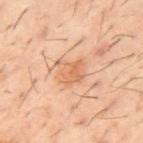biopsy status = no biopsy performed (imaged during a skin exam)
TBP lesion metrics = an automated nevus-likeness rating near 20 out of 100 and lesion-presence confidence of about 100/100
subject = male, in their 60s
acquisition = total-body-photography crop, ~15 mm field of view
diameter = ≈3.5 mm
body site = the mid back
illumination = cross-polarized illumination